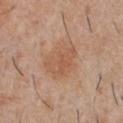Clinical impression:
This lesion was catalogued during total-body skin photography and was not selected for biopsy.
Image and clinical context:
The total-body-photography lesion software estimated a lesion area of about 12 mm² and a shape-asymmetry score of about 0.2 (0 = symmetric). It also reported a border-irregularity index near 2.5/10 and radial color variation of about 1. The software also gave a nevus-likeness score of about 35/100 and lesion-presence confidence of about 100/100. The lesion's longest dimension is about 4.5 mm. The subject is a male about 30 years old. The lesion is on the chest. This image is a 15 mm lesion crop taken from a total-body photograph.